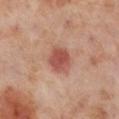Q: What did automated image analysis measure?
A: a classifier nevus-likeness of about 95/100 and lesion-presence confidence of about 100/100
Q: Who is the patient?
A: female, approximately 55 years of age
Q: Lesion size?
A: about 3.5 mm
Q: What kind of image is this?
A: ~15 mm tile from a whole-body skin photo
Q: Where on the body is the lesion?
A: the right lower leg
Q: What lighting was used for the tile?
A: cross-polarized